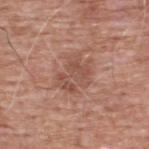| feature | finding |
|---|---|
| notes | no biopsy performed (imaged during a skin exam) |
| lighting | white-light |
| patient | male, aged 58–62 |
| site | the upper back |
| image | 15 mm crop, total-body photography |